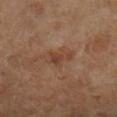Assessment:
Captured during whole-body skin photography for melanoma surveillance; the lesion was not biopsied.
Context:
This is a cross-polarized tile. From the left lower leg. Cropped from a whole-body photographic skin survey; the tile spans about 15 mm. About 3 mm across. A female patient about 70 years old.